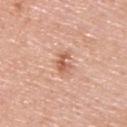Impression: Imaged during a routine full-body skin examination; the lesion was not biopsied and no histopathology is available. Acquisition and patient details: The lesion is located on the upper back. Cropped from a whole-body photographic skin survey; the tile spans about 15 mm. The subject is a male aged 53–57.